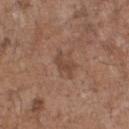No biopsy was performed on this lesion — it was imaged during a full skin examination and was not determined to be concerning.
On the chest.
Cropped from a total-body skin-imaging series; the visible field is about 15 mm.
Imaged with white-light lighting.
A male subject aged 68–72.
Measured at roughly 3 mm in maximum diameter.